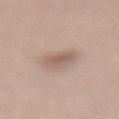The lesion was tiled from a total-body skin photograph and was not biopsied. The lesion's longest dimension is about 2.5 mm. A female patient, approximately 30 years of age. Imaged with white-light lighting. A roughly 15 mm field-of-view crop from a total-body skin photograph. From the lower back.